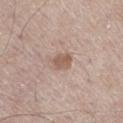follow-up: imaged on a skin check; not biopsied | illumination: white-light | diameter: about 2.5 mm | automated lesion analysis: a lesion area of about 4.5 mm², an outline eccentricity of about 0.6 (0 = round, 1 = elongated), and two-axis asymmetry of about 0.2 | patient: male, aged 63–67 | site: the right thigh | imaging modality: total-body-photography crop, ~15 mm field of view.